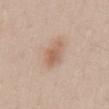biopsy status — no biopsy performed (imaged during a skin exam)
image — total-body-photography crop, ~15 mm field of view
location — the abdomen
subject — male, in their mid- to late 30s